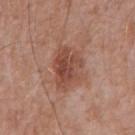{"biopsy_status": "not biopsied; imaged during a skin examination", "image": {"source": "total-body photography crop", "field_of_view_mm": 15}, "site": "back", "automated_metrics": {"cielab_L": 47, "cielab_a": 23, "cielab_b": 28, "vs_skin_contrast_norm": 7.5}, "patient": {"sex": "male", "age_approx": 60}, "lesion_size": {"long_diameter_mm_approx": 5.0}}